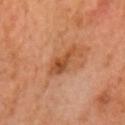– biopsy status — imaged on a skin check; not biopsied
– acquisition — 15 mm crop, total-body photography
– illumination — cross-polarized
– body site — the head or neck
– automated lesion analysis — border irregularity of about 4.5 on a 0–10 scale and internal color variation of about 3.5 on a 0–10 scale
– subject — male, roughly 60 years of age